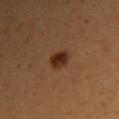The lesion was tiled from a total-body skin photograph and was not biopsied.
Located on the right upper arm.
The patient is a female aged 23–27.
A 15 mm crop from a total-body photograph taken for skin-cancer surveillance.
The recorded lesion diameter is about 2.5 mm.
Imaged with cross-polarized lighting.
Automated image analysis of the tile measured an automated nevus-likeness rating near 100 out of 100 and a lesion-detection confidence of about 100/100.